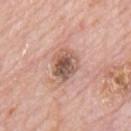Q: Is there a histopathology result?
A: total-body-photography surveillance lesion; no biopsy
Q: Illumination type?
A: white-light illumination
Q: Patient demographics?
A: male, aged around 80
Q: Lesion location?
A: the mid back
Q: Lesion size?
A: about 3.5 mm
Q: What is the imaging modality?
A: 15 mm crop, total-body photography
Q: What did automated image analysis measure?
A: an area of roughly 9 mm² and a symmetry-axis asymmetry near 0.2; a mean CIELAB color near L≈56 a*≈20 b*≈26, roughly 13 lightness units darker than nearby skin, and a lesion-to-skin contrast of about 8.5 (normalized; higher = more distinct); a border-irregularity index near 2/10, a color-variation rating of about 7/10, and radial color variation of about 2; a classifier nevus-likeness of about 0/100 and lesion-presence confidence of about 100/100